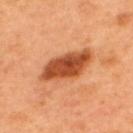notes: total-body-photography surveillance lesion; no biopsy | lesion diameter: ≈7 mm | location: the upper back | patient: male, in their 50s | acquisition: ~15 mm crop, total-body skin-cancer survey.